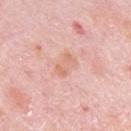Assessment:
Captured during whole-body skin photography for melanoma surveillance; the lesion was not biopsied.
Clinical summary:
On the arm. Cropped from a whole-body photographic skin survey; the tile spans about 15 mm. Measured at roughly 3 mm in maximum diameter. Imaged with white-light lighting. A female subject in their mid-60s.